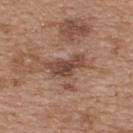On the upper back. Measured at roughly 4.5 mm in maximum diameter. Automated image analysis of the tile measured a border-irregularity index near 5/10, internal color variation of about 3 on a 0–10 scale, and radial color variation of about 1. The subject is a female in their 40s. A lesion tile, about 15 mm wide, cut from a 3D total-body photograph.